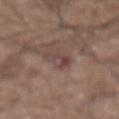| key | value |
|---|---|
| workup | total-body-photography surveillance lesion; no biopsy |
| body site | the abdomen |
| imaging modality | total-body-photography crop, ~15 mm field of view |
| subject | male, aged 73–77 |
| automated metrics | a lesion area of about 5 mm²; an automated nevus-likeness rating near 0 out of 100 and lesion-presence confidence of about 65/100 |